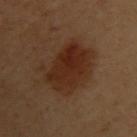biopsy status = imaged on a skin check; not biopsied | patient = female, aged 58–62 | acquisition = 15 mm crop, total-body photography | anatomic site = the upper back.